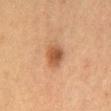Assessment:
Recorded during total-body skin imaging; not selected for excision or biopsy.
Acquisition and patient details:
Imaged with cross-polarized lighting. The lesion is located on the chest. Cropped from a whole-body photographic skin survey; the tile spans about 15 mm. About 3 mm across. A female subject about 50 years old.